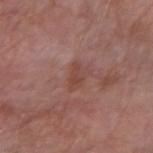The lesion was tiled from a total-body skin photograph and was not biopsied.
Approximately 3 mm at its widest.
From the right forearm.
Automated image analysis of the tile measured an average lesion color of about L≈45 a*≈23 b*≈25 (CIELAB), roughly 7 lightness units darker than nearby skin, and a normalized lesion–skin contrast near 6. And it measured a border-irregularity rating of about 3.5/10 and radial color variation of about 1. The analysis additionally found an automated nevus-likeness rating near 0 out of 100 and a lesion-detection confidence of about 100/100.
The subject is a female aged around 65.
Cropped from a whole-body photographic skin survey; the tile spans about 15 mm.